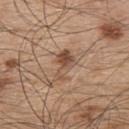Q: Was a biopsy performed?
A: catalogued during a skin exam; not biopsied
Q: What kind of image is this?
A: total-body-photography crop, ~15 mm field of view
Q: Lesion size?
A: ~3.5 mm (longest diameter)
Q: Patient demographics?
A: male, aged approximately 50
Q: Illumination type?
A: white-light
Q: Where on the body is the lesion?
A: the upper back